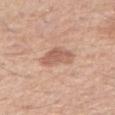No biopsy was performed on this lesion — it was imaged during a full skin examination and was not determined to be concerning.
The lesion is located on the right upper arm.
Longest diameter approximately 3.5 mm.
This image is a 15 mm lesion crop taken from a total-body photograph.
Automated image analysis of the tile measured a lesion area of about 8.5 mm² and a symmetry-axis asymmetry near 0.3. The analysis additionally found a lesion color around L≈60 a*≈22 b*≈29 in CIELAB, a lesion–skin lightness drop of about 10, and a normalized border contrast of about 6.5. It also reported a border-irregularity rating of about 3/10, internal color variation of about 2.5 on a 0–10 scale, and radial color variation of about 1.
The patient is a male aged approximately 60.
The tile uses white-light illumination.